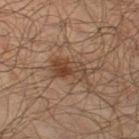Q: Was a biopsy performed?
A: imaged on a skin check; not biopsied
Q: Automated lesion metrics?
A: a lesion area of about 8.5 mm², an outline eccentricity of about 0.85 (0 = round, 1 = elongated), and a symmetry-axis asymmetry near 0.3; an average lesion color of about L≈40 a*≈17 b*≈27 (CIELAB) and a normalized border contrast of about 7.5
Q: Where on the body is the lesion?
A: the left thigh
Q: Who is the patient?
A: male, roughly 65 years of age
Q: How was the tile lit?
A: cross-polarized
Q: How large is the lesion?
A: ~4.5 mm (longest diameter)
Q: How was this image acquired?
A: ~15 mm crop, total-body skin-cancer survey